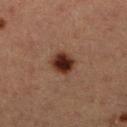Assessment:
Recorded during total-body skin imaging; not selected for excision or biopsy.
Context:
Captured under cross-polarized illumination. A female subject aged 38 to 42. This image is a 15 mm lesion crop taken from a total-body photograph. The lesion is on the right lower leg. An algorithmic analysis of the crop reported a mean CIELAB color near L≈24 a*≈19 b*≈22, about 14 CIELAB-L* units darker than the surrounding skin, and a normalized lesion–skin contrast near 14. The recorded lesion diameter is about 3 mm.